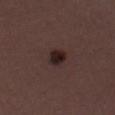Impression:
Recorded during total-body skin imaging; not selected for excision or biopsy.
Image and clinical context:
The tile uses white-light illumination. The lesion's longest dimension is about 2.5 mm. Located on the chest. The subject is a male aged around 30. A 15 mm close-up tile from a total-body photography series done for melanoma screening.